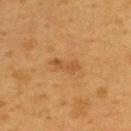| field | value |
|---|---|
| biopsy status | total-body-photography surveillance lesion; no biopsy |
| subject | male, in their mid-50s |
| site | the back |
| lighting | cross-polarized illumination |
| size | ≈3.5 mm |
| image | 15 mm crop, total-body photography |
| automated lesion analysis | a footprint of about 4 mm² and a shape-asymmetry score of about 0.4 (0 = symmetric); a border-irregularity index near 5/10, internal color variation of about 0.5 on a 0–10 scale, and radial color variation of about 0; a classifier nevus-likeness of about 5/100 and lesion-presence confidence of about 100/100 |